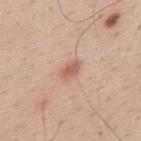workup = imaged on a skin check; not biopsied
lesion size = about 2.5 mm
image-analysis metrics = a border-irregularity index near 3/10, a color-variation rating of about 1.5/10, and peripheral color asymmetry of about 0.5; a classifier nevus-likeness of about 80/100 and a detector confidence of about 100 out of 100 that the crop contains a lesion
illumination = white-light
image source = ~15 mm crop, total-body skin-cancer survey
subject = male, roughly 40 years of age
site = the back